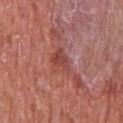{
  "biopsy_status": "not biopsied; imaged during a skin examination",
  "image": {
    "source": "total-body photography crop",
    "field_of_view_mm": 15
  },
  "automated_metrics": {
    "cielab_L": 46,
    "cielab_a": 28,
    "cielab_b": 26,
    "vs_skin_darker_L": 8.0,
    "vs_skin_contrast_norm": 6.5,
    "border_irregularity_0_10": 4.0,
    "peripheral_color_asymmetry": 1.0
  },
  "site": "chest",
  "lesion_size": {
    "long_diameter_mm_approx": 4.0
  },
  "patient": {
    "sex": "male",
    "age_approx": 65
  },
  "lighting": "white-light"
}